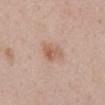Imaged during a routine full-body skin examination; the lesion was not biopsied and no histopathology is available. A female patient, about 40 years old. A 15 mm close-up tile from a total-body photography series done for melanoma screening. The lesion-visualizer software estimated a lesion area of about 5.5 mm² and a shape-asymmetry score of about 0.3 (0 = symmetric). The software also gave a lesion color around L≈59 a*≈20 b*≈29 in CIELAB, about 9 CIELAB-L* units darker than the surrounding skin, and a normalized border contrast of about 6.5. The lesion is located on the mid back. Longest diameter approximately 3 mm. The tile uses white-light illumination.